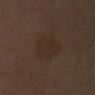Clinical impression:
Imaged during a routine full-body skin examination; the lesion was not biopsied and no histopathology is available.
Background:
A female subject, aged around 55. The lesion's longest dimension is about 4.5 mm. From the right upper arm. An algorithmic analysis of the crop reported two-axis asymmetry of about 0.25. The software also gave about 4 CIELAB-L* units darker than the surrounding skin and a normalized border contrast of about 5. The analysis additionally found a border-irregularity index near 3/10 and a within-lesion color-variation index near 1.5/10. Cropped from a total-body skin-imaging series; the visible field is about 15 mm.